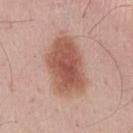Q: Was this lesion biopsied?
A: imaged on a skin check; not biopsied
Q: How large is the lesion?
A: ≈7.5 mm
Q: Patient demographics?
A: male, in their mid- to late 30s
Q: Where on the body is the lesion?
A: the mid back
Q: What is the imaging modality?
A: 15 mm crop, total-body photography
Q: How was the tile lit?
A: white-light
Q: Automated lesion metrics?
A: an area of roughly 25 mm² and an eccentricity of roughly 0.8; a lesion color around L≈55 a*≈23 b*≈28 in CIELAB, about 13 CIELAB-L* units darker than the surrounding skin, and a lesion-to-skin contrast of about 9 (normalized; higher = more distinct); a border-irregularity rating of about 2/10, internal color variation of about 4.5 on a 0–10 scale, and peripheral color asymmetry of about 1.5; a classifier nevus-likeness of about 100/100